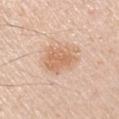follow-up: no biopsy performed (imaged during a skin exam) | site: the left upper arm | imaging modality: total-body-photography crop, ~15 mm field of view | tile lighting: white-light illumination | patient: male, approximately 60 years of age.